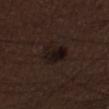The tile uses white-light illumination.
The lesion is on the leg.
The patient is a male aged around 30.
A close-up tile cropped from a whole-body skin photograph, about 15 mm across.
An algorithmic analysis of the crop reported a classifier nevus-likeness of about 25/100 and a detector confidence of about 100 out of 100 that the crop contains a lesion.